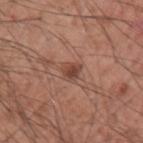Part of a total-body skin-imaging series; this lesion was reviewed on a skin check and was not flagged for biopsy. The patient is a male aged 58–62. The lesion is located on the left upper arm. Cropped from a whole-body photographic skin survey; the tile spans about 15 mm. Approximately 2.5 mm at its widest. The tile uses white-light illumination.